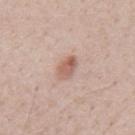The lesion's longest dimension is about 3 mm. The patient is a male aged around 40. A 15 mm crop from a total-body photograph taken for skin-cancer surveillance. Captured under white-light illumination. The lesion is located on the back. The lesion-visualizer software estimated a nevus-likeness score of about 85/100 and lesion-presence confidence of about 100/100.